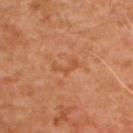| key | value |
|---|---|
| biopsy status | no biopsy performed (imaged during a skin exam) |
| body site | the upper back |
| patient | male, aged 48–52 |
| lesion diameter | about 3 mm |
| acquisition | ~15 mm tile from a whole-body skin photo |
| automated metrics | an average lesion color of about L≈52 a*≈26 b*≈39 (CIELAB) and a normalized border contrast of about 5; a lesion-detection confidence of about 100/100 |